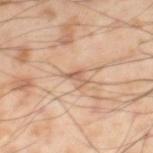The lesion was tiled from a total-body skin photograph and was not biopsied.
The lesion is located on the left thigh.
Cropped from a whole-body photographic skin survey; the tile spans about 15 mm.
The patient is a male roughly 55 years of age.